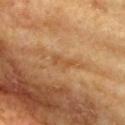Clinical impression: Part of a total-body skin-imaging series; this lesion was reviewed on a skin check and was not flagged for biopsy. Image and clinical context: The lesion is on the chest. About 3 mm across. The patient is a female about 75 years old. This is a cross-polarized tile. A close-up tile cropped from a whole-body skin photograph, about 15 mm across.